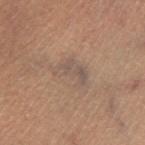workup = catalogued during a skin exam; not biopsied | site = the left lower leg | patient = male, about 50 years old | image source = ~15 mm tile from a whole-body skin photo.